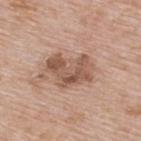No biopsy was performed on this lesion — it was imaged during a full skin examination and was not determined to be concerning. The recorded lesion diameter is about 5.5 mm. Automated image analysis of the tile measured a lesion area of about 15 mm², an outline eccentricity of about 0.75 (0 = round, 1 = elongated), and two-axis asymmetry of about 0.35. The subject is a male about 65 years old. A close-up tile cropped from a whole-body skin photograph, about 15 mm across. Located on the upper back. Imaged with white-light lighting.